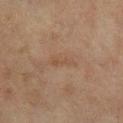Imaged during a routine full-body skin examination; the lesion was not biopsied and no histopathology is available. Imaged with cross-polarized lighting. A 15 mm crop from a total-body photograph taken for skin-cancer surveillance. The total-body-photography lesion software estimated a lesion area of about 2.5 mm², a shape eccentricity near 0.95, and a symmetry-axis asymmetry near 0.35. Measured at roughly 3 mm in maximum diameter. A female subject aged 53–57. The lesion is located on the leg.